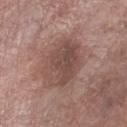Case summary:
- workup: catalogued during a skin exam; not biopsied